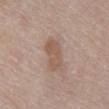{"biopsy_status": "not biopsied; imaged during a skin examination", "lighting": "white-light", "automated_metrics": {"eccentricity": 0.85, "shape_asymmetry": 0.2, "border_irregularity_0_10": 2.5, "color_variation_0_10": 2.0, "nevus_likeness_0_100": 15, "lesion_detection_confidence_0_100": 100}, "site": "abdomen", "image": {"source": "total-body photography crop", "field_of_view_mm": 15}, "patient": {"sex": "male", "age_approx": 80}, "lesion_size": {"long_diameter_mm_approx": 4.0}}